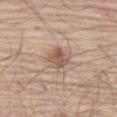Q: Was this lesion biopsied?
A: imaged on a skin check; not biopsied
Q: Lesion location?
A: the leg
Q: How was this image acquired?
A: ~15 mm tile from a whole-body skin photo
Q: What did automated image analysis measure?
A: a lesion area of about 6 mm²; a border-irregularity rating of about 2.5/10, a within-lesion color-variation index near 4.5/10, and radial color variation of about 1.5
Q: Patient demographics?
A: male, in their 80s
Q: Illumination type?
A: white-light illumination
Q: Lesion size?
A: about 2.5 mm